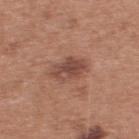Findings:
• follow-up · total-body-photography surveillance lesion; no biopsy
• body site · the upper back
• subject · male, in their mid-50s
• illumination · white-light illumination
• diameter · about 4 mm
• image source · 15 mm crop, total-body photography